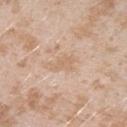Q: Was a biopsy performed?
A: imaged on a skin check; not biopsied
Q: Who is the patient?
A: female, aged 23–27
Q: Lesion location?
A: the arm
Q: How was the tile lit?
A: white-light illumination
Q: What is the imaging modality?
A: total-body-photography crop, ~15 mm field of view